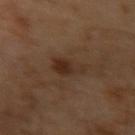Case summary:
* biopsy status: catalogued during a skin exam; not biopsied
* imaging modality: ~15 mm crop, total-body skin-cancer survey
* illumination: cross-polarized illumination
* lesion size: ≈4.5 mm
* patient: male, aged approximately 65